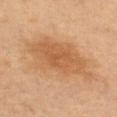Imaged during a routine full-body skin examination; the lesion was not biopsied and no histopathology is available. On the left thigh. A roughly 15 mm field-of-view crop from a total-body skin photograph. A female patient, approximately 55 years of age.